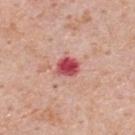* biopsy status: imaged on a skin check; not biopsied
* anatomic site: the upper back
* tile lighting: white-light
* image: ~15 mm crop, total-body skin-cancer survey
* subject: male, aged 58–62